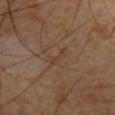Impression: No biopsy was performed on this lesion — it was imaged during a full skin examination and was not determined to be concerning. Image and clinical context: A roughly 15 mm field-of-view crop from a total-body skin photograph. Imaged with cross-polarized lighting. On the arm. The total-body-photography lesion software estimated an outline eccentricity of about 0.95 (0 = round, 1 = elongated) and two-axis asymmetry of about 0.45. The software also gave a lesion color around L≈38 a*≈15 b*≈26 in CIELAB. It also reported border irregularity of about 5 on a 0–10 scale, a within-lesion color-variation index near 0/10, and a peripheral color-asymmetry measure near 0. The recorded lesion diameter is about 3.5 mm. A male subject aged 58 to 62.